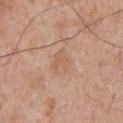Clinical impression:
The lesion was tiled from a total-body skin photograph and was not biopsied.
Clinical summary:
The total-body-photography lesion software estimated a mean CIELAB color near L≈59 a*≈19 b*≈32, roughly 6 lightness units darker than nearby skin, and a normalized border contrast of about 4.5. And it measured an automated nevus-likeness rating near 0 out of 100 and lesion-presence confidence of about 100/100. A 15 mm crop from a total-body photograph taken for skin-cancer surveillance. A male subject, aged approximately 55. Longest diameter approximately 3 mm. On the upper back.